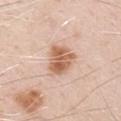Context:
Automated image analysis of the tile measured a mean CIELAB color near L≈62 a*≈21 b*≈32, a lesion–skin lightness drop of about 13, and a lesion-to-skin contrast of about 9 (normalized; higher = more distinct). The software also gave a border-irregularity rating of about 2.5/10, internal color variation of about 5 on a 0–10 scale, and radial color variation of about 1.5. It also reported a classifier nevus-likeness of about 95/100 and a detector confidence of about 100 out of 100 that the crop contains a lesion. On the left upper arm. The patient is a male about 70 years old. Approximately 4 mm at its widest. The tile uses white-light illumination. A 15 mm close-up tile from a total-body photography series done for melanoma screening.